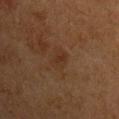<case>
<biopsy_status>not biopsied; imaged during a skin examination</biopsy_status>
<patient>
  <sex>male</sex>
  <age_approx>75</age_approx>
</patient>
<image>
  <source>total-body photography crop</source>
  <field_of_view_mm>15</field_of_view_mm>
</image>
<site>chest</site>
<automated_metrics>
  <eccentricity>0.65</eccentricity>
  <shape_asymmetry>0.2</shape_asymmetry>
  <border_irregularity_0_10>2.0</border_irregularity_0_10>
  <lesion_detection_confidence_0_100>100</lesion_detection_confidence_0_100>
</automated_metrics>
<lighting>cross-polarized</lighting>
</case>